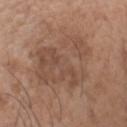workup — total-body-photography surveillance lesion; no biopsy | illumination — white-light illumination | diameter — ~9 mm (longest diameter) | anatomic site — the head or neck | imaging modality — ~15 mm crop, total-body skin-cancer survey | subject — male, about 60 years old.